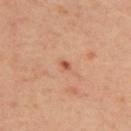follow-up: total-body-photography surveillance lesion; no biopsy
body site: the upper back
image: ~15 mm tile from a whole-body skin photo
TBP lesion metrics: a footprint of about 1 mm², an outline eccentricity of about 0.75 (0 = round, 1 = elongated), and two-axis asymmetry of about 0.45; an average lesion color of about L≈51 a*≈28 b*≈34 (CIELAB), about 12 CIELAB-L* units darker than the surrounding skin, and a normalized lesion–skin contrast near 8.5; border irregularity of about 3 on a 0–10 scale, a within-lesion color-variation index near 0/10, and peripheral color asymmetry of about 0; lesion-presence confidence of about 100/100
size: ~1.5 mm (longest diameter)
subject: female, approximately 45 years of age
tile lighting: cross-polarized